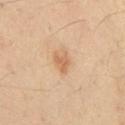Case summary:
• imaging modality · ~15 mm crop, total-body skin-cancer survey
• patient · male, aged approximately 60
• location · the abdomen